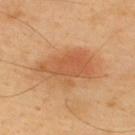The lesion was photographed on a routine skin check and not biopsied; there is no pathology result. The lesion is on the upper back. An algorithmic analysis of the crop reported a footprint of about 19 mm². The software also gave a lesion color around L≈57 a*≈24 b*≈39 in CIELAB, about 10 CIELAB-L* units darker than the surrounding skin, and a normalized border contrast of about 6.5. The software also gave a border-irregularity index near 3.5/10, a within-lesion color-variation index near 5/10, and a peripheral color-asymmetry measure near 2. Captured under cross-polarized illumination. A male patient, about 40 years old. About 6.5 mm across. A close-up tile cropped from a whole-body skin photograph, about 15 mm across.